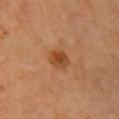biopsy status — no biopsy performed (imaged during a skin exam).